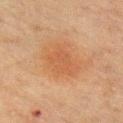{"biopsy_status": "not biopsied; imaged during a skin examination", "lighting": "cross-polarized", "site": "left upper arm", "lesion_size": {"long_diameter_mm_approx": 5.0}, "automated_metrics": {"cielab_L": 46, "cielab_a": 20, "cielab_b": 32, "vs_skin_darker_L": 6.0, "vs_skin_contrast_norm": 5.0, "border_irregularity_0_10": 3.0, "color_variation_0_10": 2.0, "peripheral_color_asymmetry": 0.5, "lesion_detection_confidence_0_100": 100}, "patient": {"sex": "male", "age_approx": 65}, "image": {"source": "total-body photography crop", "field_of_view_mm": 15}}